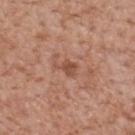Part of a total-body skin-imaging series; this lesion was reviewed on a skin check and was not flagged for biopsy. Automated tile analysis of the lesion measured border irregularity of about 5.5 on a 0–10 scale and a color-variation rating of about 1.5/10. A lesion tile, about 15 mm wide, cut from a 3D total-body photograph. From the mid back. Imaged with white-light lighting. The patient is a male approximately 65 years of age.